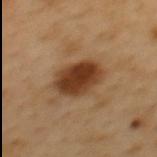No biopsy was performed on this lesion — it was imaged during a full skin examination and was not determined to be concerning. Located on the mid back. The patient is a male aged around 50. Imaged with cross-polarized lighting. Cropped from a whole-body photographic skin survey; the tile spans about 15 mm. Longest diameter approximately 5 mm.